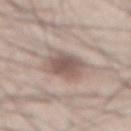The lesion was photographed on a routine skin check and not biopsied; there is no pathology result.